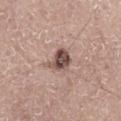automated_metrics:
  area_mm2_approx: 7.0
  eccentricity: 0.65
  shape_asymmetry: 0.35
  vs_skin_contrast_norm: 10.5
  border_irregularity_0_10: 3.5
  color_variation_0_10: 7.0
  peripheral_color_asymmetry: 2.5
  nevus_likeness_0_100: 85
patient:
  sex: male
  age_approx: 70
lighting: white-light
site: right thigh
lesion_size:
  long_diameter_mm_approx: 4.0
image:
  source: total-body photography crop
  field_of_view_mm: 15